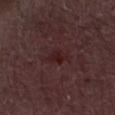Assessment: The lesion was tiled from a total-body skin photograph and was not biopsied. Clinical summary: The subject is a male roughly 50 years of age. The tile uses white-light illumination. A lesion tile, about 15 mm wide, cut from a 3D total-body photograph.